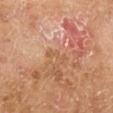Imaged during a routine full-body skin examination; the lesion was not biopsied and no histopathology is available. The lesion is located on the right lower leg. Cropped from a total-body skin-imaging series; the visible field is about 15 mm. The lesion-visualizer software estimated an automated nevus-likeness rating near 0 out of 100. A male patient, aged 63 to 67.